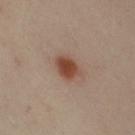This lesion was catalogued during total-body skin photography and was not selected for biopsy.
The total-body-photography lesion software estimated a lesion color around L≈45 a*≈22 b*≈29 in CIELAB, about 13 CIELAB-L* units darker than the surrounding skin, and a normalized border contrast of about 10.
This image is a 15 mm lesion crop taken from a total-body photograph.
Captured under cross-polarized illumination.
The lesion is on the left arm.
The lesion's longest dimension is about 3 mm.
The subject is a female aged around 40.